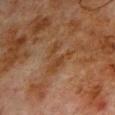Imaged during a routine full-body skin examination; the lesion was not biopsied and no histopathology is available. Approximately 4.5 mm at its widest. The lesion-visualizer software estimated a lesion–skin lightness drop of about 5 and a lesion-to-skin contrast of about 6 (normalized; higher = more distinct). It also reported a within-lesion color-variation index near 1/10 and a peripheral color-asymmetry measure near 0. And it measured a nevus-likeness score of about 0/100. Cropped from a whole-body photographic skin survey; the tile spans about 15 mm. Captured under cross-polarized illumination. On the upper back. The patient is a male aged 78–82.